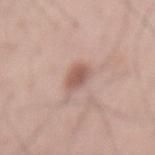Image and clinical context:
The total-body-photography lesion software estimated a lesion color around L≈56 a*≈21 b*≈25 in CIELAB. The software also gave a border-irregularity rating of about 1.5/10, a color-variation rating of about 2/10, and peripheral color asymmetry of about 0.5. The software also gave a classifier nevus-likeness of about 80/100. This is a white-light tile. Longest diameter approximately 2.5 mm. A 15 mm close-up tile from a total-body photography series done for melanoma screening. A male subject, aged 68 to 72. The lesion is located on the mid back.